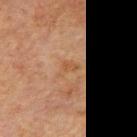Recorded during total-body skin imaging; not selected for excision or biopsy. On the left arm. A male subject, about 60 years old. The recorded lesion diameter is about 3 mm. This is a cross-polarized tile. A roughly 15 mm field-of-view crop from a total-body skin photograph.